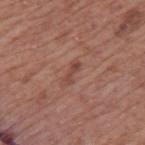<case>
  <biopsy_status>not biopsied; imaged during a skin examination</biopsy_status>
  <automated_metrics>
    <cielab_L>45</cielab_L>
    <cielab_a>23</cielab_a>
    <cielab_b>25</cielab_b>
    <vs_skin_darker_L>8.0</vs_skin_darker_L>
    <vs_skin_contrast_norm>6.5</vs_skin_contrast_norm>
    <border_irregularity_0_10>4.0</border_irregularity_0_10>
    <color_variation_0_10>0.0</color_variation_0_10>
    <peripheral_color_asymmetry>0.0</peripheral_color_asymmetry>
    <lesion_detection_confidence_0_100>100</lesion_detection_confidence_0_100>
  </automated_metrics>
  <image>
    <source>total-body photography crop</source>
    <field_of_view_mm>15</field_of_view_mm>
  </image>
  <lighting>white-light</lighting>
  <site>mid back</site>
  <patient>
    <sex>male</sex>
    <age_approx>75</age_approx>
  </patient>
</case>